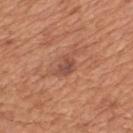No biopsy was performed on this lesion — it was imaged during a full skin examination and was not determined to be concerning. From the mid back. Longest diameter approximately 2.5 mm. Cropped from a whole-body photographic skin survey; the tile spans about 15 mm. The subject is a male aged 63–67. The lesion-visualizer software estimated a lesion area of about 4 mm² and an eccentricity of roughly 0.65. It also reported a lesion–skin lightness drop of about 9. The software also gave a border-irregularity index near 3/10, a color-variation rating of about 3/10, and a peripheral color-asymmetry measure near 1. Captured under white-light illumination.